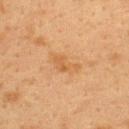{
  "biopsy_status": "not biopsied; imaged during a skin examination",
  "lighting": "cross-polarized",
  "site": "upper back",
  "image": {
    "source": "total-body photography crop",
    "field_of_view_mm": 15
  },
  "patient": {
    "sex": "female",
    "age_approx": 40
  },
  "automated_metrics": {
    "nevus_likeness_0_100": 0,
    "lesion_detection_confidence_0_100": 100
  }
}